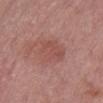location: the front of the torso | image: ~15 mm tile from a whole-body skin photo | patient: male, aged 73 to 77 | TBP lesion metrics: a footprint of about 10 mm², a shape eccentricity near 0.75, and a shape-asymmetry score of about 0.2 (0 = symmetric); lesion-presence confidence of about 100/100.